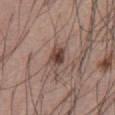This lesion was catalogued during total-body skin photography and was not selected for biopsy. Captured under white-light illumination. The recorded lesion diameter is about 4 mm. The total-body-photography lesion software estimated an area of roughly 7 mm². It also reported border irregularity of about 3 on a 0–10 scale, a within-lesion color-variation index near 6.5/10, and radial color variation of about 2. The lesion is located on the abdomen. A close-up tile cropped from a whole-body skin photograph, about 15 mm across. A male subject, roughly 55 years of age.